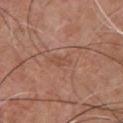Q: Was a biopsy performed?
A: catalogued during a skin exam; not biopsied
Q: What kind of image is this?
A: 15 mm crop, total-body photography
Q: What is the lesion's diameter?
A: about 2.5 mm
Q: Automated lesion metrics?
A: a lesion area of about 2.5 mm², an outline eccentricity of about 0.9 (0 = round, 1 = elongated), and a shape-asymmetry score of about 0.3 (0 = symmetric); a lesion color around L≈49 a*≈22 b*≈29 in CIELAB and a normalized border contrast of about 4.5; an automated nevus-likeness rating near 0 out of 100
Q: What is the anatomic site?
A: the chest
Q: Patient demographics?
A: male, approximately 55 years of age